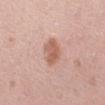The lesion was photographed on a routine skin check and not biopsied; there is no pathology result. The lesion is located on the right forearm. The patient is a female aged 38–42. This is a white-light tile. An algorithmic analysis of the crop reported an area of roughly 7 mm², an outline eccentricity of about 0.5 (0 = round, 1 = elongated), and a symmetry-axis asymmetry near 0.2. The software also gave a lesion–skin lightness drop of about 10 and a lesion-to-skin contrast of about 7 (normalized; higher = more distinct). And it measured border irregularity of about 2 on a 0–10 scale, internal color variation of about 3 on a 0–10 scale, and peripheral color asymmetry of about 1. A 15 mm close-up extracted from a 3D total-body photography capture.